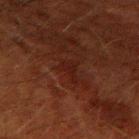Recorded during total-body skin imaging; not selected for excision or biopsy. The recorded lesion diameter is about 2.5 mm. On the right upper arm. A 15 mm close-up tile from a total-body photography series done for melanoma screening. A male patient, approximately 50 years of age.